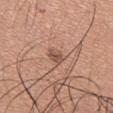biopsy status=catalogued during a skin exam; not biopsied | body site=the right upper arm | patient=male, aged approximately 35 | image=15 mm crop, total-body photography.